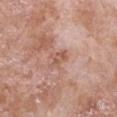<lesion>
  <biopsy_status>not biopsied; imaged during a skin examination</biopsy_status>
  <lighting>white-light</lighting>
  <site>front of the torso</site>
  <lesion_size>
    <long_diameter_mm_approx>3.0</long_diameter_mm_approx>
  </lesion_size>
  <patient>
    <sex>female</sex>
    <age_approx>75</age_approx>
  </patient>
  <image>
    <source>total-body photography crop</source>
    <field_of_view_mm>15</field_of_view_mm>
  </image>
</lesion>